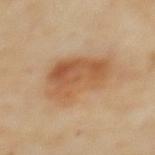This lesion was catalogued during total-body skin photography and was not selected for biopsy. The patient is a female about 45 years old. The tile uses cross-polarized illumination. From the upper back. Cropped from a total-body skin-imaging series; the visible field is about 15 mm. Approximately 7.5 mm at its widest.